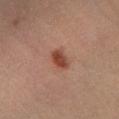Clinical impression: Recorded during total-body skin imaging; not selected for excision or biopsy. Image and clinical context: This image is a 15 mm lesion crop taken from a total-body photograph. The lesion is on the right lower leg. The recorded lesion diameter is about 3 mm. Captured under cross-polarized illumination. A male patient aged 38–42.